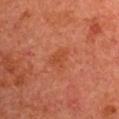notes = no biopsy performed (imaged during a skin exam)
size = about 3 mm
subject = male, aged 63–67
imaging modality = ~15 mm tile from a whole-body skin photo
image-analysis metrics = a footprint of about 5.5 mm², a shape eccentricity near 0.7, and a symmetry-axis asymmetry near 0.4; border irregularity of about 3.5 on a 0–10 scale, a color-variation rating of about 1.5/10, and peripheral color asymmetry of about 0.5; a classifier nevus-likeness of about 0/100 and a detector confidence of about 100 out of 100 that the crop contains a lesion
site = the right upper arm
lighting = cross-polarized illumination